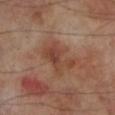No biopsy was performed on this lesion — it was imaged during a full skin examination and was not determined to be concerning. The lesion is on the left lower leg. Cropped from a total-body skin-imaging series; the visible field is about 15 mm. A male subject, aged approximately 70.